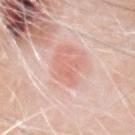Recorded during total-body skin imaging; not selected for excision or biopsy. On the head or neck. Imaged with white-light lighting. A close-up tile cropped from a whole-body skin photograph, about 15 mm across. An algorithmic analysis of the crop reported a lesion color around L≈67 a*≈26 b*≈28 in CIELAB and roughly 8 lightness units darker than nearby skin. It also reported a classifier nevus-likeness of about 100/100 and a detector confidence of about 100 out of 100 that the crop contains a lesion. Approximately 3.5 mm at its widest. A male patient in their 80s.